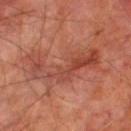Image and clinical context:
A 15 mm crop from a total-body photograph taken for skin-cancer surveillance. A male patient, aged 68–72. From the leg.